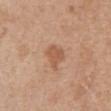notes: imaged on a skin check; not biopsied | image source: ~15 mm crop, total-body skin-cancer survey | patient: female, aged 73–77 | automated metrics: a footprint of about 5 mm² and an outline eccentricity of about 0.55 (0 = round, 1 = elongated); a lesion color around L≈56 a*≈22 b*≈33 in CIELAB and a lesion–skin lightness drop of about 9; an automated nevus-likeness rating near 25 out of 100 and a lesion-detection confidence of about 100/100 | site: the chest.